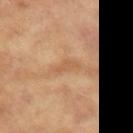| field | value |
|---|---|
| workup | total-body-photography surveillance lesion; no biopsy |
| image source | ~15 mm tile from a whole-body skin photo |
| patient | female, aged 73–77 |
| location | the arm |
| size | ≈3 mm |